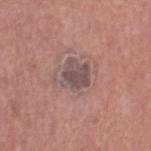Captured during whole-body skin photography for melanoma surveillance; the lesion was not biopsied.
The lesion's longest dimension is about 3 mm.
Located on the right thigh.
A female subject, about 50 years old.
This is a white-light tile.
This image is a 15 mm lesion crop taken from a total-body photograph.